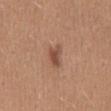The lesion was tiled from a total-body skin photograph and was not biopsied. Located on the mid back. Captured under white-light illumination. A 15 mm close-up extracted from a 3D total-body photography capture. The recorded lesion diameter is about 3 mm. The subject is a female aged around 40.